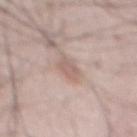Q: Was a biopsy performed?
A: total-body-photography surveillance lesion; no biopsy
Q: What is the lesion's diameter?
A: ~3 mm (longest diameter)
Q: What are the patient's age and sex?
A: male, roughly 50 years of age
Q: Lesion location?
A: the abdomen
Q: Illumination type?
A: white-light illumination
Q: What is the imaging modality?
A: 15 mm crop, total-body photography